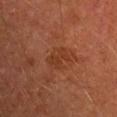Q: Was this lesion biopsied?
A: no biopsy performed (imaged during a skin exam)
Q: What is the imaging modality?
A: 15 mm crop, total-body photography
Q: Where on the body is the lesion?
A: the right upper arm
Q: Lesion size?
A: ≈3 mm
Q: Who is the patient?
A: male, about 65 years old
Q: How was the tile lit?
A: cross-polarized
Q: What did automated image analysis measure?
A: an area of roughly 6 mm², an eccentricity of roughly 0.7, and a shape-asymmetry score of about 0.3 (0 = symmetric); border irregularity of about 3.5 on a 0–10 scale, a within-lesion color-variation index near 2.5/10, and radial color variation of about 1